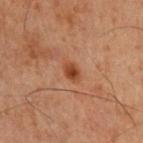Case summary:
– follow-up — total-body-photography surveillance lesion; no biopsy
– tile lighting — cross-polarized
– size — about 2.5 mm
– imaging modality — ~15 mm crop, total-body skin-cancer survey
– automated metrics — an area of roughly 4 mm², an outline eccentricity of about 0.75 (0 = round, 1 = elongated), and a symmetry-axis asymmetry near 0.25; border irregularity of about 2 on a 0–10 scale, a color-variation rating of about 3.5/10, and a peripheral color-asymmetry measure near 1
– location — the right upper arm
– subject — male, about 60 years old